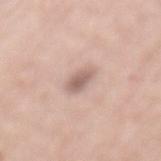<case>
<biopsy_status>not biopsied; imaged during a skin examination</biopsy_status>
<lesion_size>
  <long_diameter_mm_approx>3.5</long_diameter_mm_approx>
</lesion_size>
<site>mid back</site>
<lighting>white-light</lighting>
<image>
  <source>total-body photography crop</source>
  <field_of_view_mm>15</field_of_view_mm>
</image>
<patient>
  <sex>female</sex>
  <age_approx>60</age_approx>
</patient>
<automated_metrics>
  <eccentricity>0.8</eccentricity>
  <shape_asymmetry>0.2</shape_asymmetry>
  <cielab_L>62</cielab_L>
  <cielab_a>17</cielab_a>
  <cielab_b>24</cielab_b>
  <vs_skin_darker_L>11.0</vs_skin_darker_L>
  <vs_skin_contrast_norm>7.0</vs_skin_contrast_norm>
  <border_irregularity_0_10>2.0</border_irregularity_0_10>
  <peripheral_color_asymmetry>1.0</peripheral_color_asymmetry>
</automated_metrics>
</case>